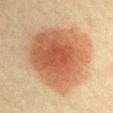Part of a total-body skin-imaging series; this lesion was reviewed on a skin check and was not flagged for biopsy.
The tile uses cross-polarized illumination.
A male subject aged 38 to 42.
The lesion is on the left upper arm.
A 15 mm crop from a total-body photograph taken for skin-cancer surveillance.
Automated tile analysis of the lesion measured an eccentricity of roughly 0.5 and two-axis asymmetry of about 0.2. And it measured a nevus-likeness score of about 100/100.